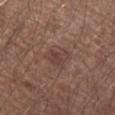Imaged during a routine full-body skin examination; the lesion was not biopsied and no histopathology is available. A male patient, in their mid-50s. A lesion tile, about 15 mm wide, cut from a 3D total-body photograph. Captured under white-light illumination. The total-body-photography lesion software estimated a footprint of about 5 mm², a shape eccentricity near 0.75, and a symmetry-axis asymmetry near 0.4. It also reported an automated nevus-likeness rating near 0 out of 100 and a detector confidence of about 100 out of 100 that the crop contains a lesion. On the arm. About 3 mm across.